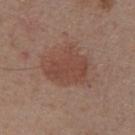Notes:
– workup · imaged on a skin check; not biopsied
– anatomic site · the arm
– diameter · about 5.5 mm
– image source · ~15 mm tile from a whole-body skin photo
– tile lighting · white-light
– subject · male, approximately 55 years of age